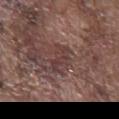Impression: Imaged during a routine full-body skin examination; the lesion was not biopsied and no histopathology is available. Image and clinical context: Located on the front of the torso. The patient is a male aged around 75. A region of skin cropped from a whole-body photographic capture, roughly 15 mm wide.